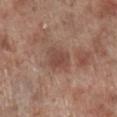Q: What is the imaging modality?
A: ~15 mm crop, total-body skin-cancer survey
Q: Lesion location?
A: the right lower leg
Q: What are the patient's age and sex?
A: male, aged around 70
Q: What did automated image analysis measure?
A: a footprint of about 6 mm², a shape eccentricity near 0.6, and a shape-asymmetry score of about 0.25 (0 = symmetric); a mean CIELAB color near L≈46 a*≈21 b*≈25, a lesion–skin lightness drop of about 8, and a normalized border contrast of about 6.5; a border-irregularity rating of about 2.5/10 and internal color variation of about 3 on a 0–10 scale; a nevus-likeness score of about 10/100 and a lesion-detection confidence of about 100/100